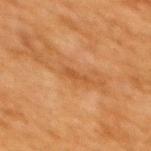Findings:
• follow-up — total-body-photography surveillance lesion; no biopsy
• tile lighting — cross-polarized illumination
• subject — female, about 55 years old
• image source — 15 mm crop, total-body photography
• diameter — ~2.5 mm (longest diameter)
• anatomic site — the upper back